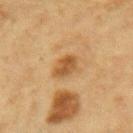follow-up — no biopsy performed (imaged during a skin exam)
anatomic site — the right forearm
tile lighting — cross-polarized
subject — female, in their mid-40s
image source — ~15 mm tile from a whole-body skin photo